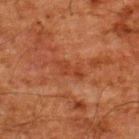The patient is a male aged around 60.
Cropped from a whole-body photographic skin survey; the tile spans about 15 mm.
The lesion-visualizer software estimated a lesion area of about 4.5 mm² and an outline eccentricity of about 0.95 (0 = round, 1 = elongated). The analysis additionally found an average lesion color of about L≈32 a*≈24 b*≈30 (CIELAB) and a lesion–skin lightness drop of about 6. The software also gave internal color variation of about 1 on a 0–10 scale and a peripheral color-asymmetry measure near 0. It also reported a nevus-likeness score of about 0/100 and lesion-presence confidence of about 60/100.
Approximately 4 mm at its widest.
The lesion is on the upper back.
Captured under cross-polarized illumination.